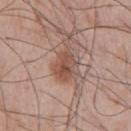The lesion was photographed on a routine skin check and not biopsied; there is no pathology result. From the chest. Measured at roughly 4.5 mm in maximum diameter. The tile uses white-light illumination. A 15 mm crop from a total-body photograph taken for skin-cancer surveillance. A male subject aged approximately 65.